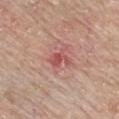A roughly 15 mm field-of-view crop from a total-body skin photograph.
On the chest.
Imaged with white-light lighting.
An algorithmic analysis of the crop reported a lesion color around L≈53 a*≈29 b*≈25 in CIELAB, a lesion–skin lightness drop of about 10, and a lesion-to-skin contrast of about 6.5 (normalized; higher = more distinct).
A male patient aged 78–82.
The recorded lesion diameter is about 2.5 mm.